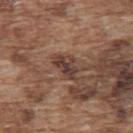biopsy_status: not biopsied; imaged during a skin examination
site: upper back
image:
  source: total-body photography crop
  field_of_view_mm: 15
automated_metrics:
  area_mm2_approx: 5.0
  eccentricity: 0.8
  shape_asymmetry: 0.4
patient:
  sex: male
  age_approx: 75